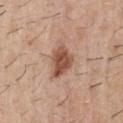Clinical impression:
Imaged during a routine full-body skin examination; the lesion was not biopsied and no histopathology is available.
Background:
A male patient aged approximately 30. Automated image analysis of the tile measured a footprint of about 9 mm² and a shape-asymmetry score of about 0.25 (0 = symmetric). It also reported border irregularity of about 2.5 on a 0–10 scale and a peripheral color-asymmetry measure near 1.5. A roughly 15 mm field-of-view crop from a total-body skin photograph. Located on the chest. The lesion's longest dimension is about 4 mm. The tile uses white-light illumination.